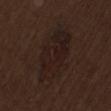Q: Is there a histopathology result?
A: no biopsy performed (imaged during a skin exam)
Q: What did automated image analysis measure?
A: a border-irregularity rating of about 3.5/10 and radial color variation of about 1.5
Q: What is the anatomic site?
A: the lower back
Q: How was this image acquired?
A: ~15 mm tile from a whole-body skin photo
Q: Who is the patient?
A: male, aged 68–72